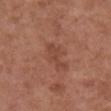Imaged during a routine full-body skin examination; the lesion was not biopsied and no histopathology is available.
A lesion tile, about 15 mm wide, cut from a 3D total-body photograph.
A female patient, aged approximately 75.
The lesion is located on the chest.
Approximately 4 mm at its widest.